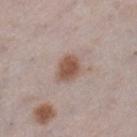Recorded during total-body skin imaging; not selected for excision or biopsy.